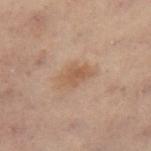Recorded during total-body skin imaging; not selected for excision or biopsy. The lesion is located on the left leg. A lesion tile, about 15 mm wide, cut from a 3D total-body photograph. A female patient aged around 55.